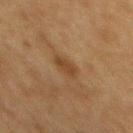| field | value |
|---|---|
| biopsy status | catalogued during a skin exam; not biopsied |
| image-analysis metrics | a lesion area of about 4.5 mm², a shape eccentricity near 0.8, and two-axis asymmetry of about 0.2; border irregularity of about 2 on a 0–10 scale, internal color variation of about 1.5 on a 0–10 scale, and a peripheral color-asymmetry measure near 0.5; a detector confidence of about 100 out of 100 that the crop contains a lesion |
| patient | male, roughly 75 years of age |
| image | ~15 mm crop, total-body skin-cancer survey |
| tile lighting | cross-polarized |
| anatomic site | the mid back |
| lesion diameter | ≈3 mm |